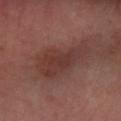Context:
An algorithmic analysis of the crop reported an average lesion color of about L≈35 a*≈21 b*≈22 (CIELAB), roughly 8 lightness units darker than nearby skin, and a normalized lesion–skin contrast near 7. It also reported border irregularity of about 5 on a 0–10 scale, internal color variation of about 3.5 on a 0–10 scale, and peripheral color asymmetry of about 1. The analysis additionally found a classifier nevus-likeness of about 5/100 and a lesion-detection confidence of about 100/100. The tile uses cross-polarized illumination. Cropped from a whole-body photographic skin survey; the tile spans about 15 mm. From the left forearm. A male subject, aged 48 to 52. Measured at roughly 7 mm in maximum diameter.